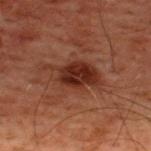{
  "image": {
    "source": "total-body photography crop",
    "field_of_view_mm": 15
  },
  "lighting": "cross-polarized",
  "patient": {
    "sex": "male",
    "age_approx": 60
  },
  "site": "upper back",
  "lesion_size": {
    "long_diameter_mm_approx": 6.0
  },
  "automated_metrics": {
    "border_irregularity_0_10": 3.5,
    "color_variation_0_10": 4.5,
    "peripheral_color_asymmetry": 1.5,
    "nevus_likeness_0_100": 80,
    "lesion_detection_confidence_0_100": 100
  }
}